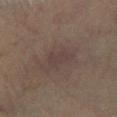<lesion>
<biopsy_status>not biopsied; imaged during a skin examination</biopsy_status>
<patient>
  <sex>male</sex>
  <age_approx>65</age_approx>
</patient>
<lesion_size>
  <long_diameter_mm_approx>3.0</long_diameter_mm_approx>
</lesion_size>
<lighting>cross-polarized</lighting>
<automated_metrics>
  <eccentricity>0.7</eccentricity>
  <cielab_L>37</cielab_L>
  <cielab_a>14</cielab_a>
  <cielab_b>17</cielab_b>
  <vs_skin_darker_L>4.0</vs_skin_darker_L>
</automated_metrics>
<image>
  <source>total-body photography crop</source>
  <field_of_view_mm>15</field_of_view_mm>
</image>
</lesion>